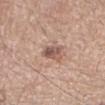Clinical impression: The lesion was tiled from a total-body skin photograph and was not biopsied. Acquisition and patient details: On the right forearm. The patient is a male aged around 60. The recorded lesion diameter is about 3 mm. A roughly 15 mm field-of-view crop from a total-body skin photograph. The lesion-visualizer software estimated a footprint of about 5 mm² and two-axis asymmetry of about 0.2. And it measured a lesion color around L≈54 a*≈19 b*≈25 in CIELAB, about 12 CIELAB-L* units darker than the surrounding skin, and a normalized border contrast of about 8. It also reported a peripheral color-asymmetry measure near 2.5. The software also gave an automated nevus-likeness rating near 75 out of 100 and a lesion-detection confidence of about 100/100. Captured under white-light illumination.